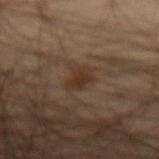* patient · male, aged 53–57
* automated metrics · roughly 6 lightness units darker than nearby skin and a normalized lesion–skin contrast near 7.5; border irregularity of about 3 on a 0–10 scale, a color-variation rating of about 1.5/10, and peripheral color asymmetry of about 0.5; a classifier nevus-likeness of about 80/100 and lesion-presence confidence of about 100/100
* lighting · cross-polarized
* image · total-body-photography crop, ~15 mm field of view
* anatomic site · the left forearm